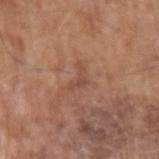The tile uses white-light illumination. The lesion is located on the left upper arm. The lesion's longest dimension is about 3 mm. The subject is a male aged approximately 75. A 15 mm close-up tile from a total-body photography series done for melanoma screening.